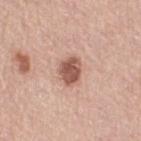Findings:
• location — the right thigh
• acquisition — total-body-photography crop, ~15 mm field of view
• subject — female, roughly 65 years of age